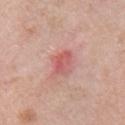No biopsy was performed on this lesion — it was imaged during a full skin examination and was not determined to be concerning.